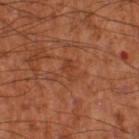This lesion was catalogued during total-body skin photography and was not selected for biopsy. Longest diameter approximately 2.5 mm. The lesion-visualizer software estimated a nevus-likeness score of about 0/100 and lesion-presence confidence of about 100/100. The lesion is on the left thigh. A lesion tile, about 15 mm wide, cut from a 3D total-body photograph. A male subject roughly 55 years of age. The tile uses cross-polarized illumination.